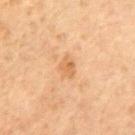<lesion>
  <biopsy_status>not biopsied; imaged during a skin examination</biopsy_status>
  <lesion_size>
    <long_diameter_mm_approx>3.0</long_diameter_mm_approx>
  </lesion_size>
  <automated_metrics>
    <area_mm2_approx>4.5</area_mm2_approx>
    <eccentricity>0.7</eccentricity>
    <shape_asymmetry>0.3</shape_asymmetry>
  </automated_metrics>
  <patient>
    <sex>female</sex>
    <age_approx>80</age_approx>
  </patient>
  <site>upper back</site>
  <image>
    <source>total-body photography crop</source>
    <field_of_view_mm>15</field_of_view_mm>
  </image>
  <lighting>cross-polarized</lighting>
</lesion>